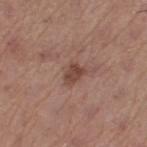Recorded during total-body skin imaging; not selected for excision or biopsy. Approximately 2.5 mm at its widest. The lesion is on the left thigh. A female subject aged 63 to 67. An algorithmic analysis of the crop reported border irregularity of about 3.5 on a 0–10 scale. And it measured a nevus-likeness score of about 60/100 and a lesion-detection confidence of about 100/100. A roughly 15 mm field-of-view crop from a total-body skin photograph.